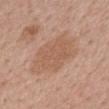notes = total-body-photography surveillance lesion; no biopsy
site = the mid back
lesion diameter = about 7 mm
image = 15 mm crop, total-body photography
subject = male, in their mid- to late 50s
illumination = white-light illumination